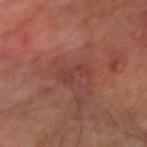Clinical impression:
Part of a total-body skin-imaging series; this lesion was reviewed on a skin check and was not flagged for biopsy.
Image and clinical context:
Located on the right forearm. The total-body-photography lesion software estimated a lesion area of about 3.5 mm² and a symmetry-axis asymmetry near 0.5. And it measured a lesion-detection confidence of about 100/100. This is a cross-polarized tile. A roughly 15 mm field-of-view crop from a total-body skin photograph. The lesion's longest dimension is about 3.5 mm.